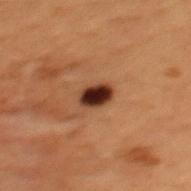Assessment:
The lesion was photographed on a routine skin check and not biopsied; there is no pathology result.
Acquisition and patient details:
A region of skin cropped from a whole-body photographic capture, roughly 15 mm wide. A male patient, approximately 50 years of age. Located on the mid back.